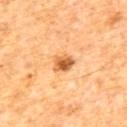Clinical impression: Recorded during total-body skin imaging; not selected for excision or biopsy. Clinical summary: The subject is a male in their mid-60s. Automated tile analysis of the lesion measured a footprint of about 4.5 mm² and two-axis asymmetry of about 0.2. And it measured a nevus-likeness score of about 95/100. Approximately 2.5 mm at its widest. Located on the mid back. Cropped from a whole-body photographic skin survey; the tile spans about 15 mm.